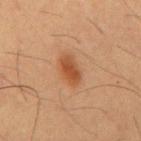Part of a total-body skin-imaging series; this lesion was reviewed on a skin check and was not flagged for biopsy. The patient is a male in their mid- to late 50s. A lesion tile, about 15 mm wide, cut from a 3D total-body photograph. The lesion is located on the chest.